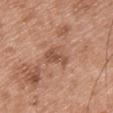Part of a total-body skin-imaging series; this lesion was reviewed on a skin check and was not flagged for biopsy. A male subject, aged 48 to 52. The lesion is located on the chest. Cropped from a total-body skin-imaging series; the visible field is about 15 mm.